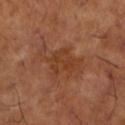Assessment:
The lesion was photographed on a routine skin check and not biopsied; there is no pathology result.
Background:
The total-body-photography lesion software estimated a lesion area of about 13 mm², a shape eccentricity near 0.85, and a shape-asymmetry score of about 0.35 (0 = symmetric). The analysis additionally found a lesion color around L≈39 a*≈23 b*≈33 in CIELAB and a lesion-to-skin contrast of about 6 (normalized; higher = more distinct). And it measured a border-irregularity index near 7/10, internal color variation of about 2.5 on a 0–10 scale, and a peripheral color-asymmetry measure near 0.5. The software also gave an automated nevus-likeness rating near 0 out of 100 and a lesion-detection confidence of about 100/100. The tile uses cross-polarized illumination. A male subject in their mid- to late 60s. From the left lower leg. Cropped from a total-body skin-imaging series; the visible field is about 15 mm.